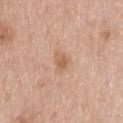biopsy_status: not biopsied; imaged during a skin examination
lesion_size:
  long_diameter_mm_approx: 2.5
site: upper back
patient:
  sex: male
  age_approx: 40
image:
  source: total-body photography crop
  field_of_view_mm: 15
lighting: white-light